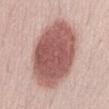Q: Was this lesion biopsied?
A: no biopsy performed (imaged during a skin exam)
Q: What are the patient's age and sex?
A: female, about 45 years old
Q: Lesion location?
A: the abdomen
Q: How was the tile lit?
A: white-light
Q: What kind of image is this?
A: ~15 mm crop, total-body skin-cancer survey